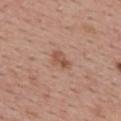Clinical impression: Recorded during total-body skin imaging; not selected for excision or biopsy. Background: This image is a 15 mm lesion crop taken from a total-body photograph. On the upper back. Automated tile analysis of the lesion measured an outline eccentricity of about 0.85 (0 = round, 1 = elongated) and a symmetry-axis asymmetry near 0.3. The tile uses white-light illumination. The patient is a female approximately 55 years of age.